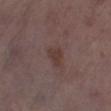| field | value |
|---|---|
| workup | no biopsy performed (imaged during a skin exam) |
| patient | male, in their mid- to late 70s |
| body site | the right lower leg |
| tile lighting | white-light |
| imaging modality | ~15 mm tile from a whole-body skin photo |